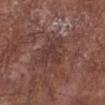Notes:
– follow-up: imaged on a skin check; not biopsied